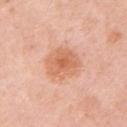Case summary:
* workup — imaged on a skin check; not biopsied
* automated lesion analysis — an area of roughly 12 mm², an eccentricity of roughly 0.6, and two-axis asymmetry of about 0.15
* acquisition — total-body-photography crop, ~15 mm field of view
* subject — female, approximately 50 years of age
* size — ~4.5 mm (longest diameter)
* illumination — white-light
* anatomic site — the left upper arm